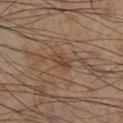Assessment: This lesion was catalogued during total-body skin photography and was not selected for biopsy. Clinical summary: A male subject, in their mid- to late 50s. Measured at roughly 3 mm in maximum diameter. Captured under cross-polarized illumination. Cropped from a total-body skin-imaging series; the visible field is about 15 mm. An algorithmic analysis of the crop reported a shape-asymmetry score of about 0.3 (0 = symmetric). And it measured a mean CIELAB color near L≈43 a*≈16 b*≈29, a lesion–skin lightness drop of about 7, and a lesion-to-skin contrast of about 5.5 (normalized; higher = more distinct). And it measured internal color variation of about 2 on a 0–10 scale and peripheral color asymmetry of about 0.5. The lesion is on the right lower leg.